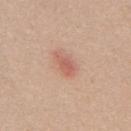Assessment:
The lesion was tiled from a total-body skin photograph and was not biopsied.
Clinical summary:
A region of skin cropped from a whole-body photographic capture, roughly 15 mm wide. The patient is a male roughly 30 years of age. This is a white-light tile. From the upper back. Measured at roughly 3 mm in maximum diameter.